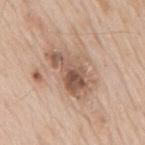biopsy_status: not biopsied; imaged during a skin examination
patient:
  sex: male
  age_approx: 65
image:
  source: total-body photography crop
  field_of_view_mm: 15
site: mid back